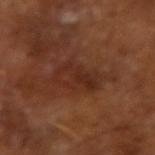notes: imaged on a skin check; not biopsied | subject: male, aged approximately 65 | TBP lesion metrics: an area of roughly 11 mm², a shape eccentricity near 0.85, and two-axis asymmetry of about 0.2; an automated nevus-likeness rating near 0 out of 100 and lesion-presence confidence of about 100/100 | size: ≈5 mm | tile lighting: cross-polarized | imaging modality: ~15 mm tile from a whole-body skin photo.